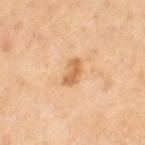{
  "biopsy_status": "not biopsied; imaged during a skin examination",
  "patient": {
    "sex": "female",
    "age_approx": 55
  },
  "lighting": "cross-polarized",
  "lesion_size": {
    "long_diameter_mm_approx": 3.0
  },
  "site": "leg",
  "image": {
    "source": "total-body photography crop",
    "field_of_view_mm": 15
  }
}